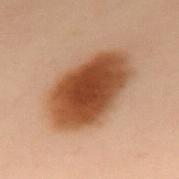Q: Was this lesion biopsied?
A: no biopsy performed (imaged during a skin exam)